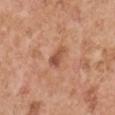TBP lesion metrics = a symmetry-axis asymmetry near 0.35
patient = male, roughly 55 years of age
lesion diameter = ≈2.5 mm
body site = the right upper arm
lighting = white-light
image source = total-body-photography crop, ~15 mm field of view